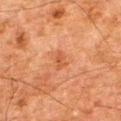| feature | finding |
|---|---|
| workup | no biopsy performed (imaged during a skin exam) |
| imaging modality | total-body-photography crop, ~15 mm field of view |
| subject | male, aged 78–82 |
| lesion diameter | about 2.5 mm |
| tile lighting | cross-polarized |
| body site | the right thigh |